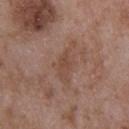A male subject aged 48–52.
Cropped from a whole-body photographic skin survey; the tile spans about 15 mm.
Approximately 3 mm at its widest.
Automated image analysis of the tile measured an area of roughly 3.5 mm² and an eccentricity of roughly 0.85. The analysis additionally found an average lesion color of about L≈46 a*≈19 b*≈27 (CIELAB). And it measured border irregularity of about 3.5 on a 0–10 scale, a within-lesion color-variation index near 0.5/10, and radial color variation of about 0.
From the back.
This is a white-light tile.